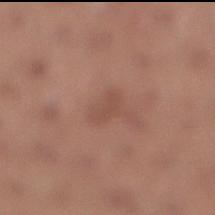Clinical impression:
Imaged during a routine full-body skin examination; the lesion was not biopsied and no histopathology is available.
Image and clinical context:
The recorded lesion diameter is about 2.5 mm. The patient is a female aged 63 to 67. A close-up tile cropped from a whole-body skin photograph, about 15 mm across. Imaged with white-light lighting. The lesion is located on the leg.